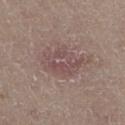| field | value |
|---|---|
| biopsy status | imaged on a skin check; not biopsied |
| anatomic site | the right thigh |
| image | total-body-photography crop, ~15 mm field of view |
| patient | male, aged around 75 |
| size | ≈6.5 mm |